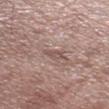follow-up: no biopsy performed (imaged during a skin exam)